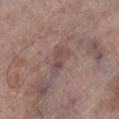The lesion is on the leg. Imaged with white-light lighting. A roughly 15 mm field-of-view crop from a total-body skin photograph. The lesion-visualizer software estimated a lesion area of about 5 mm², a shape eccentricity near 0.9, and two-axis asymmetry of about 0.4. The software also gave a border-irregularity index near 4.5/10 and radial color variation of about 2. The patient is a male about 70 years old. About 3.5 mm across.